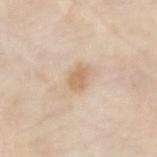Notes:
* lesion size: ~2.5 mm (longest diameter)
* anatomic site: the left forearm
* image source: total-body-photography crop, ~15 mm field of view
* patient: male, aged 78–82